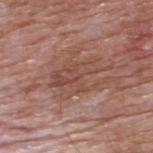workup: imaged on a skin check; not biopsied | anatomic site: the upper back | automated lesion analysis: an area of roughly 14 mm², a shape eccentricity near 0.8, and a shape-asymmetry score of about 0.45 (0 = symmetric); an average lesion color of about L≈48 a*≈21 b*≈26 (CIELAB) and a normalized border contrast of about 5.5 | subject: male, about 60 years old | size: about 6.5 mm | acquisition: ~15 mm tile from a whole-body skin photo.